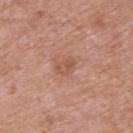Captured during whole-body skin photography for melanoma surveillance; the lesion was not biopsied. This image is a 15 mm lesion crop taken from a total-body photograph. The recorded lesion diameter is about 2.5 mm. A female patient, about 40 years old. This is a white-light tile. The lesion is on the upper back. The total-body-photography lesion software estimated a lesion color around L≈54 a*≈23 b*≈29 in CIELAB and a normalized border contrast of about 5.5. The analysis additionally found a color-variation rating of about 3/10 and a peripheral color-asymmetry measure near 1. The analysis additionally found a detector confidence of about 100 out of 100 that the crop contains a lesion.